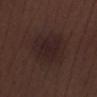Q: Was a biopsy performed?
A: catalogued during a skin exam; not biopsied